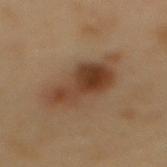{"image": {"source": "total-body photography crop", "field_of_view_mm": 15}, "site": "mid back", "lighting": "cross-polarized", "lesion_size": {"long_diameter_mm_approx": 8.5}, "patient": {"sex": "male", "age_approx": 55}, "automated_metrics": {"eccentricity": 0.9, "shape_asymmetry": 0.25}}